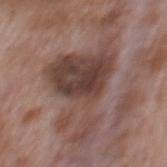{"biopsy_status": "not biopsied; imaged during a skin examination", "site": "mid back", "patient": {"sex": "male", "age_approx": 70}, "automated_metrics": {"eccentricity": 0.7, "shape_asymmetry": 0.65, "vs_skin_darker_L": 12.0, "vs_skin_contrast_norm": 9.0, "border_irregularity_0_10": 9.0, "color_variation_0_10": 5.0, "peripheral_color_asymmetry": 1.0}, "image": {"source": "total-body photography crop", "field_of_view_mm": 15}}